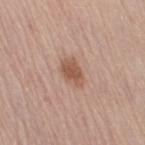Imaged during a routine full-body skin examination; the lesion was not biopsied and no histopathology is available. A 15 mm crop from a total-body photograph taken for skin-cancer surveillance. The patient is a female aged approximately 65. The lesion is located on the right thigh. This is a white-light tile.